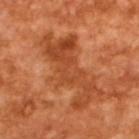Clinical impression:
The lesion was photographed on a routine skin check and not biopsied; there is no pathology result.
Background:
Cropped from a whole-body photographic skin survey; the tile spans about 15 mm. Captured under cross-polarized illumination. About 9 mm across. The patient is a male in their mid-60s. Automated image analysis of the tile measured a nevus-likeness score of about 0/100 and a detector confidence of about 100 out of 100 that the crop contains a lesion.